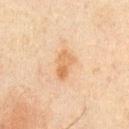The total-body-photography lesion software estimated a nevus-likeness score of about 30/100 and a lesion-detection confidence of about 100/100. A male subject, about 45 years old. Imaged with cross-polarized lighting. A region of skin cropped from a whole-body photographic capture, roughly 15 mm wide. From the chest.